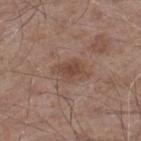– workup — catalogued during a skin exam; not biopsied
– acquisition — 15 mm crop, total-body photography
– location — the right lower leg
– automated metrics — an area of roughly 6.5 mm², an outline eccentricity of about 0.8 (0 = round, 1 = elongated), and two-axis asymmetry of about 0.3; a border-irregularity rating of about 3.5/10, internal color variation of about 2.5 on a 0–10 scale, and a peripheral color-asymmetry measure near 1
– diameter — ≈3.5 mm
– patient — male, roughly 55 years of age